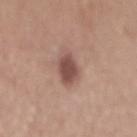{
  "biopsy_status": "not biopsied; imaged during a skin examination",
  "image": {
    "source": "total-body photography crop",
    "field_of_view_mm": 15
  },
  "patient": {
    "sex": "male",
    "age_approx": 50
  },
  "site": "mid back"
}